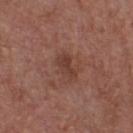follow-up: imaged on a skin check; not biopsied | patient: male, about 55 years old | anatomic site: the chest | lesion size: ~3 mm (longest diameter) | imaging modality: total-body-photography crop, ~15 mm field of view.